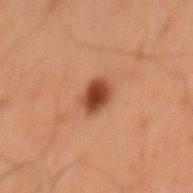The lesion was photographed on a routine skin check and not biopsied; there is no pathology result. On the mid back. A male subject aged 58 to 62. A 15 mm close-up extracted from a 3D total-body photography capture. Imaged with cross-polarized lighting. About 3 mm across.